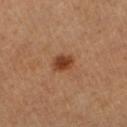Clinical impression: This lesion was catalogued during total-body skin photography and was not selected for biopsy. Context: The lesion is on the right upper arm. A close-up tile cropped from a whole-body skin photograph, about 15 mm across. A male subject roughly 60 years of age.